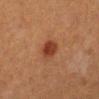{
  "biopsy_status": "not biopsied; imaged during a skin examination",
  "site": "left lower leg",
  "automated_metrics": {
    "area_mm2_approx": 6.0,
    "eccentricity": 0.6,
    "shape_asymmetry": 0.2,
    "cielab_L": 34,
    "cielab_a": 24,
    "cielab_b": 29,
    "vs_skin_darker_L": 10.0,
    "vs_skin_contrast_norm": 8.5,
    "color_variation_0_10": 2.5,
    "peripheral_color_asymmetry": 1.0,
    "lesion_detection_confidence_0_100": 100
  },
  "lesion_size": {
    "long_diameter_mm_approx": 3.0
  },
  "patient": {
    "sex": "female",
    "age_approx": 55
  },
  "lighting": "cross-polarized",
  "image": {
    "source": "total-body photography crop",
    "field_of_view_mm": 15
  }
}